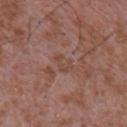Captured during whole-body skin photography for melanoma surveillance; the lesion was not biopsied.